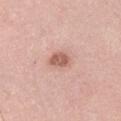| feature | finding |
|---|---|
| follow-up | imaged on a skin check; not biopsied |
| patient | male, roughly 40 years of age |
| imaging modality | 15 mm crop, total-body photography |
| tile lighting | white-light illumination |
| location | the right upper arm |